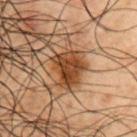Recorded during total-body skin imaging; not selected for excision or biopsy.
From the right upper arm.
Cropped from a total-body skin-imaging series; the visible field is about 15 mm.
The subject is a male about 50 years old.
This is a cross-polarized tile.
Longest diameter approximately 4 mm.
Automated image analysis of the tile measured a lesion area of about 11 mm², an eccentricity of roughly 0.55, and two-axis asymmetry of about 0.35. The analysis additionally found a lesion color around L≈32 a*≈18 b*≈28 in CIELAB and a normalized border contrast of about 11. And it measured a nevus-likeness score of about 90/100.